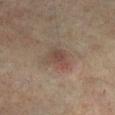<record>
  <biopsy_status>not biopsied; imaged during a skin examination</biopsy_status>
  <image>
    <source>total-body photography crop</source>
    <field_of_view_mm>15</field_of_view_mm>
  </image>
  <patient>
    <sex>male</sex>
    <age_approx>75</age_approx>
  </patient>
  <automated_metrics>
    <cielab_L>37</cielab_L>
    <cielab_a>14</cielab_a>
    <cielab_b>19</cielab_b>
    <vs_skin_darker_L>7.0</vs_skin_darker_L>
    <vs_skin_contrast_norm>6.5</vs_skin_contrast_norm>
  </automated_metrics>
  <site>right lower leg</site>
  <lesion_size>
    <long_diameter_mm_approx>3.0</long_diameter_mm_approx>
  </lesion_size>
</record>